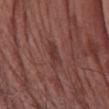Recorded during total-body skin imaging; not selected for excision or biopsy.
The lesion is located on the right forearm.
A close-up tile cropped from a whole-body skin photograph, about 15 mm across.
A male patient roughly 75 years of age.
The tile uses white-light illumination.
Approximately 3 mm at its widest.